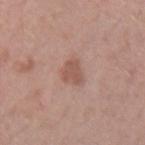Imaged with white-light lighting.
Cropped from a total-body skin-imaging series; the visible field is about 15 mm.
A male patient, in their mid-40s.
About 2.5 mm across.
The lesion is located on the right forearm.